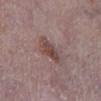biopsy status=catalogued during a skin exam; not biopsied
lighting=white-light
acquisition=~15 mm tile from a whole-body skin photo
lesion diameter=about 4 mm
anatomic site=the left lower leg
patient=male, in their mid-70s
automated lesion analysis=an automated nevus-likeness rating near 65 out of 100